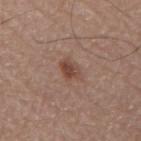{"biopsy_status": "not biopsied; imaged during a skin examination", "image": {"source": "total-body photography crop", "field_of_view_mm": 15}, "site": "mid back", "lesion_size": {"long_diameter_mm_approx": 2.5}, "lighting": "white-light", "patient": {"sex": "male", "age_approx": 80}}